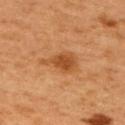  biopsy_status: not biopsied; imaged during a skin examination
  image:
    source: total-body photography crop
    field_of_view_mm: 15
  site: upper back
  patient:
    sex: male
    age_approx: 50